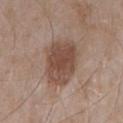Q: Is there a histopathology result?
A: total-body-photography surveillance lesion; no biopsy
Q: Automated lesion metrics?
A: an outline eccentricity of about 0.65 (0 = round, 1 = elongated) and a symmetry-axis asymmetry near 0.15; a lesion color around L≈48 a*≈18 b*≈25 in CIELAB and a lesion–skin lightness drop of about 11; an automated nevus-likeness rating near 60 out of 100 and a detector confidence of about 100 out of 100 that the crop contains a lesion
Q: What is the lesion's diameter?
A: ≈5.5 mm
Q: What are the patient's age and sex?
A: male, aged approximately 55
Q: Where on the body is the lesion?
A: the right upper arm
Q: Illumination type?
A: white-light
Q: What is the imaging modality?
A: total-body-photography crop, ~15 mm field of view